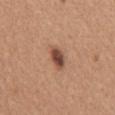illumination: white-light | imaging modality: total-body-photography crop, ~15 mm field of view | anatomic site: the mid back | TBP lesion metrics: a lesion area of about 5 mm², a shape eccentricity near 0.85, and a symmetry-axis asymmetry near 0.3; a lesion color around L≈48 a*≈22 b*≈28 in CIELAB and a normalized border contrast of about 10.5; a border-irregularity rating of about 2.5/10, a within-lesion color-variation index near 5/10, and a peripheral color-asymmetry measure near 1.5 | lesion size: about 3.5 mm | patient: female, approximately 40 years of age.